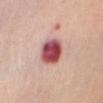Clinical impression:
Recorded during total-body skin imaging; not selected for excision or biopsy.
Acquisition and patient details:
A 15 mm crop from a total-body photograph taken for skin-cancer surveillance. The lesion-visualizer software estimated an area of roughly 10 mm², a shape eccentricity near 0.6, and two-axis asymmetry of about 0.1. It also reported border irregularity of about 1.5 on a 0–10 scale, internal color variation of about 8 on a 0–10 scale, and peripheral color asymmetry of about 2.5. Approximately 4 mm at its widest. From the chest. Captured under white-light illumination. The subject is a female approximately 65 years of age.